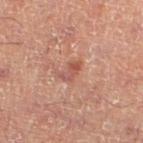biopsy status: no biopsy performed (imaged during a skin exam)
subject: male, aged approximately 50
lesion size: ~2.5 mm (longest diameter)
tile lighting: cross-polarized
image: ~15 mm crop, total-body skin-cancer survey
site: the left lower leg
automated lesion analysis: a mean CIELAB color near L≈46 a*≈23 b*≈25, about 8 CIELAB-L* units darker than the surrounding skin, and a lesion-to-skin contrast of about 6.5 (normalized; higher = more distinct)